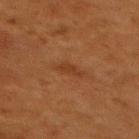The lesion is on the mid back. The lesion's longest dimension is about 2.5 mm. A female subject, roughly 50 years of age. Automated image analysis of the tile measured border irregularity of about 4 on a 0–10 scale and a color-variation rating of about 0.5/10. And it measured an automated nevus-likeness rating near 0 out of 100. Captured under cross-polarized illumination. A 15 mm close-up tile from a total-body photography series done for melanoma screening.